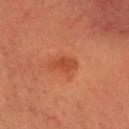workup: no biopsy performed (imaged during a skin exam) | tile lighting: cross-polarized illumination | patient: male, in their mid- to late 30s | body site: the head or neck | imaging modality: total-body-photography crop, ~15 mm field of view.